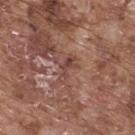The lesion is on the back.
A roughly 15 mm field-of-view crop from a total-body skin photograph.
A male patient, aged approximately 75.